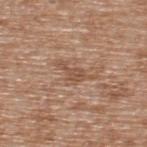notes: catalogued during a skin exam; not biopsied | subject: male, aged around 65 | anatomic site: the upper back | automated metrics: a footprint of about 5.5 mm² and a shape eccentricity near 0.85; a color-variation rating of about 2.5/10 and peripheral color asymmetry of about 1 | lighting: white-light | image: total-body-photography crop, ~15 mm field of view | lesion size: ~4.5 mm (longest diameter).